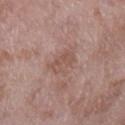Clinical impression: The lesion was photographed on a routine skin check and not biopsied; there is no pathology result. Acquisition and patient details: Located on the left lower leg. The subject is a female about 70 years old. Approximately 3 mm at its widest. Imaged with white-light lighting. A lesion tile, about 15 mm wide, cut from a 3D total-body photograph.